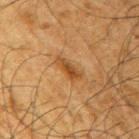Notes:
• location: the arm
• patient: male, aged 63–67
• imaging modality: 15 mm crop, total-body photography